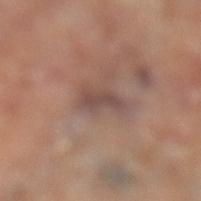Recorded during total-body skin imaging; not selected for excision or biopsy.
A roughly 15 mm field-of-view crop from a total-body skin photograph.
Automated tile analysis of the lesion measured a lesion area of about 5 mm² and an eccentricity of roughly 0.85. And it measured a mean CIELAB color near L≈48 a*≈18 b*≈22 and a normalized border contrast of about 6.5.
This is a cross-polarized tile.
On the left lower leg.